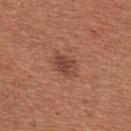No biopsy was performed on this lesion — it was imaged during a full skin examination and was not determined to be concerning.
This image is a 15 mm lesion crop taken from a total-body photograph.
The subject is a female aged around 35.
Automated tile analysis of the lesion measured border irregularity of about 2 on a 0–10 scale.
Imaged with white-light lighting.
About 3 mm across.
Located on the chest.